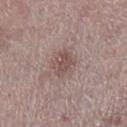workup: no biopsy performed (imaged during a skin exam) | patient: female, aged 63–67 | image: ~15 mm crop, total-body skin-cancer survey | lesion size: ~3.5 mm (longest diameter) | location: the leg | automated lesion analysis: a lesion area of about 6.5 mm², a shape eccentricity near 0.7, and a symmetry-axis asymmetry near 0.2; a border-irregularity rating of about 2.5/10 and a color-variation rating of about 2/10.